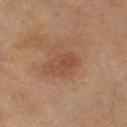notes=total-body-photography surveillance lesion; no biopsy
subject=female, roughly 60 years of age
lesion diameter=≈3 mm
image=~15 mm crop, total-body skin-cancer survey
location=the right thigh